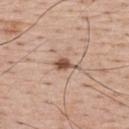{"biopsy_status": "not biopsied; imaged during a skin examination", "patient": {"sex": "male", "age_approx": 65}, "site": "back", "lighting": "white-light", "lesion_size": {"long_diameter_mm_approx": 3.5}, "image": {"source": "total-body photography crop", "field_of_view_mm": 15}}